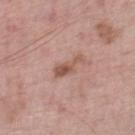Recorded during total-body skin imaging; not selected for excision or biopsy.
A male patient aged around 70.
Captured under white-light illumination.
Longest diameter approximately 3.5 mm.
A roughly 15 mm field-of-view crop from a total-body skin photograph.
From the right lower leg.